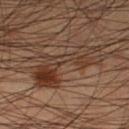{"biopsy_status": "not biopsied; imaged during a skin examination", "image": {"source": "total-body photography crop", "field_of_view_mm": 15}, "lighting": "cross-polarized", "site": "leg", "automated_metrics": {"area_mm2_approx": 20.0, "eccentricity": 0.9, "shape_asymmetry": 0.55, "cielab_L": 34, "cielab_a": 17, "cielab_b": 25, "vs_skin_darker_L": 8.0, "vs_skin_contrast_norm": 8.0, "border_irregularity_0_10": 9.0, "color_variation_0_10": 8.5, "peripheral_color_asymmetry": 2.5, "nevus_likeness_0_100": 65, "lesion_detection_confidence_0_100": 90}, "patient": {"sex": "male", "age_approx": 45}}